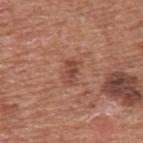follow-up = no biopsy performed (imaged during a skin exam); acquisition = total-body-photography crop, ~15 mm field of view; illumination = white-light; diameter = ~3 mm (longest diameter); patient = male, about 75 years old; location = the upper back.